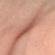{"biopsy_status": "not biopsied; imaged during a skin examination", "image": {"source": "total-body photography crop", "field_of_view_mm": 15}, "lighting": "cross-polarized", "lesion_size": {"long_diameter_mm_approx": 4.5}, "site": "arm", "patient": {"sex": "female", "age_approx": 65}}